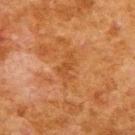Assessment:
No biopsy was performed on this lesion — it was imaged during a full skin examination and was not determined to be concerning.
Background:
Longest diameter approximately 3.5 mm. The subject is a male aged 78 to 82. From the upper back. Imaged with cross-polarized lighting. This image is a 15 mm lesion crop taken from a total-body photograph. An algorithmic analysis of the crop reported a shape eccentricity near 0.85 and a symmetry-axis asymmetry near 0.4. The software also gave border irregularity of about 5 on a 0–10 scale, a color-variation rating of about 1.5/10, and peripheral color asymmetry of about 0.5.